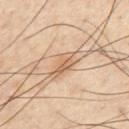notes = total-body-photography surveillance lesion; no biopsy
subject = male, approximately 45 years of age
acquisition = ~15 mm crop, total-body skin-cancer survey
site = the chest